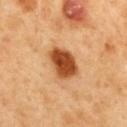Q: Was this lesion biopsied?
A: catalogued during a skin exam; not biopsied
Q: Where on the body is the lesion?
A: the mid back
Q: How large is the lesion?
A: about 4.5 mm
Q: Illumination type?
A: cross-polarized
Q: How was this image acquired?
A: total-body-photography crop, ~15 mm field of view
Q: What did automated image analysis measure?
A: an area of roughly 11 mm², an eccentricity of roughly 0.75, and two-axis asymmetry of about 0.2; a lesion color around L≈50 a*≈28 b*≈42 in CIELAB and a normalized border contrast of about 12.5; a border-irregularity rating of about 2/10 and peripheral color asymmetry of about 1.5
Q: What are the patient's age and sex?
A: male, aged approximately 50